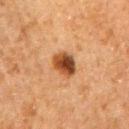Part of a total-body skin-imaging series; this lesion was reviewed on a skin check and was not flagged for biopsy. Located on the lower back. The lesion's longest dimension is about 3.5 mm. A region of skin cropped from a whole-body photographic capture, roughly 15 mm wide. Imaged with cross-polarized lighting. A male patient in their 80s.